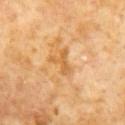notes — total-body-photography surveillance lesion; no biopsy.